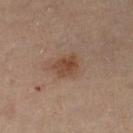Impression: The lesion was tiled from a total-body skin photograph and was not biopsied. Acquisition and patient details: On the left lower leg. A 15 mm crop from a total-body photograph taken for skin-cancer surveillance. A female patient aged around 60.